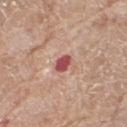No biopsy was performed on this lesion — it was imaged during a full skin examination and was not determined to be concerning.
This is a white-light tile.
A female subject, in their mid-70s.
A 15 mm close-up tile from a total-body photography series done for melanoma screening.
On the left thigh.
The recorded lesion diameter is about 2.5 mm.